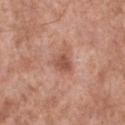Q: Is there a histopathology result?
A: total-body-photography surveillance lesion; no biopsy
Q: What is the imaging modality?
A: 15 mm crop, total-body photography
Q: How was the tile lit?
A: white-light
Q: Lesion location?
A: the left lower leg
Q: Who is the patient?
A: male, about 55 years old